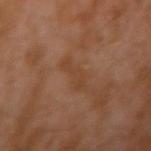  image:
    source: total-body photography crop
    field_of_view_mm: 15
  site: left arm
  lighting: cross-polarized
  patient:
    sex: male
    age_approx: 30
  lesion_size:
    long_diameter_mm_approx: 3.5